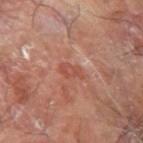follow-up = catalogued during a skin exam; not biopsied | patient = male, roughly 65 years of age | body site = the arm | diameter = about 3 mm | imaging modality = 15 mm crop, total-body photography | tile lighting = cross-polarized illumination.